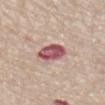biopsy_status: not biopsied; imaged during a skin examination
lesion_size:
  long_diameter_mm_approx: 3.5
patient:
  sex: male
  age_approx: 75
site: abdomen
image:
  source: total-body photography crop
  field_of_view_mm: 15
lighting: white-light
automated_metrics:
  cielab_L: 53
  cielab_a: 28
  cielab_b: 20
  vs_skin_darker_L: 17.0
  vs_skin_contrast_norm: 11.5